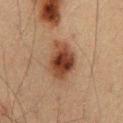Recorded during total-body skin imaging; not selected for excision or biopsy.
The patient is a male aged 58 to 62.
Located on the abdomen.
A lesion tile, about 15 mm wide, cut from a 3D total-body photograph.
Approximately 5 mm at its widest.